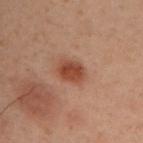Clinical impression:
Recorded during total-body skin imaging; not selected for excision or biopsy.
Background:
Longest diameter approximately 3 mm. A male patient in their mid-40s. The total-body-photography lesion software estimated a normalized lesion–skin contrast near 9. The software also gave a border-irregularity rating of about 1/10, internal color variation of about 3 on a 0–10 scale, and radial color variation of about 1. This is a cross-polarized tile. A 15 mm close-up tile from a total-body photography series done for melanoma screening. From the upper back.